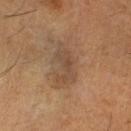– follow-up: total-body-photography surveillance lesion; no biopsy
– illumination: cross-polarized
– image: ~15 mm crop, total-body skin-cancer survey
– image-analysis metrics: an area of roughly 8.5 mm² and an eccentricity of roughly 0.9; an average lesion color of about L≈44 a*≈16 b*≈28 (CIELAB) and roughly 7 lightness units darker than nearby skin; border irregularity of about 5.5 on a 0–10 scale, a color-variation rating of about 3.5/10, and a peripheral color-asymmetry measure near 1.5
– size: ≈5 mm
– patient: male, aged approximately 60
– anatomic site: the left lower leg